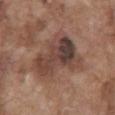Clinical impression:
Captured during whole-body skin photography for melanoma surveillance; the lesion was not biopsied.
Clinical summary:
Captured under white-light illumination. Located on the chest. A male subject aged approximately 75. Cropped from a total-body skin-imaging series; the visible field is about 15 mm.